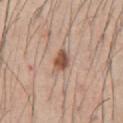This lesion was catalogued during total-body skin photography and was not selected for biopsy. A male patient in their mid-40s. This image is a 15 mm lesion crop taken from a total-body photograph. Located on the front of the torso.